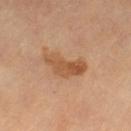  biopsy_status: not biopsied; imaged during a skin examination
  site: left thigh
  image:
    source: total-body photography crop
    field_of_view_mm: 15
  lesion_size:
    long_diameter_mm_approx: 5.0
  patient:
    sex: male
    age_approx: 65
  lighting: cross-polarized